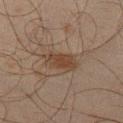| key | value |
|---|---|
| notes | catalogued during a skin exam; not biopsied |
| image | ~15 mm tile from a whole-body skin photo |
| automated lesion analysis | an outline eccentricity of about 0.85 (0 = round, 1 = elongated) and a symmetry-axis asymmetry near 0.2; an average lesion color of about L≈34 a*≈14 b*≈23 (CIELAB), a lesion–skin lightness drop of about 7, and a lesion-to-skin contrast of about 7.5 (normalized; higher = more distinct); a border-irregularity rating of about 3/10; a nevus-likeness score of about 35/100 and a lesion-detection confidence of about 100/100 |
| anatomic site | the leg |
| subject | male, aged approximately 45 |
| lighting | cross-polarized |
| lesion diameter | about 4.5 mm |